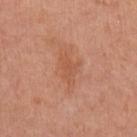| key | value |
|---|---|
| biopsy status | total-body-photography surveillance lesion; no biopsy |
| illumination | white-light |
| location | the left upper arm |
| image source | 15 mm crop, total-body photography |
| lesion diameter | ≈3 mm |
| subject | female, aged approximately 55 |